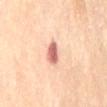Clinical impression:
Imaged during a routine full-body skin examination; the lesion was not biopsied and no histopathology is available.
Image and clinical context:
The lesion is on the back. This image is a 15 mm lesion crop taken from a total-body photograph. The recorded lesion diameter is about 2.5 mm. Automated tile analysis of the lesion measured a footprint of about 4.5 mm², an eccentricity of roughly 0.65, and two-axis asymmetry of about 0.2. The software also gave an average lesion color of about L≈66 a*≈28 b*≈28 (CIELAB), about 16 CIELAB-L* units darker than the surrounding skin, and a lesion-to-skin contrast of about 9.5 (normalized; higher = more distinct). The software also gave border irregularity of about 1.5 on a 0–10 scale, internal color variation of about 4 on a 0–10 scale, and radial color variation of about 1. The software also gave a classifier nevus-likeness of about 35/100 and a detector confidence of about 100 out of 100 that the crop contains a lesion. The patient is a female aged approximately 65. Imaged with cross-polarized lighting.